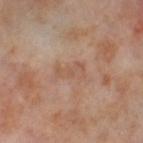<record>
  <biopsy_status>not biopsied; imaged during a skin examination</biopsy_status>
  <patient>
    <sex>female</sex>
    <age_approx>55</age_approx>
  </patient>
  <automated_metrics>
    <shape_asymmetry>0.3</shape_asymmetry>
    <cielab_L>55</cielab_L>
    <cielab_a>19</cielab_a>
    <cielab_b>29</cielab_b>
    <vs_skin_darker_L>6.0</vs_skin_darker_L>
    <vs_skin_contrast_norm>4.5</vs_skin_contrast_norm>
  </automated_metrics>
  <lighting>cross-polarized</lighting>
  <lesion_size>
    <long_diameter_mm_approx>3.5</long_diameter_mm_approx>
  </lesion_size>
  <site>leg</site>
  <image>
    <source>total-body photography crop</source>
    <field_of_view_mm>15</field_of_view_mm>
  </image>
</record>